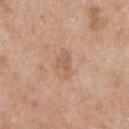Imaged during a routine full-body skin examination; the lesion was not biopsied and no histopathology is available. A lesion tile, about 15 mm wide, cut from a 3D total-body photograph. From the front of the torso. A male patient aged 48 to 52.